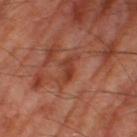The lesion was photographed on a routine skin check and not biopsied; there is no pathology result.
A male patient, aged around 70.
Measured at roughly 3.5 mm in maximum diameter.
The lesion is on the right thigh.
The tile uses cross-polarized illumination.
A close-up tile cropped from a whole-body skin photograph, about 15 mm across.